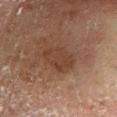{"biopsy_status": "not biopsied; imaged during a skin examination", "patient": {"sex": "male", "age_approx": 80}, "lighting": "cross-polarized", "lesion_size": {"long_diameter_mm_approx": 4.0}, "site": "left lower leg", "image": {"source": "total-body photography crop", "field_of_view_mm": 15}}